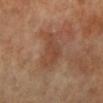The lesion was tiled from a total-body skin photograph and was not biopsied. A female patient in their mid-60s. From the leg. Approximately 4.5 mm at its widest. This is a cross-polarized tile. A 15 mm crop from a total-body photograph taken for skin-cancer surveillance.